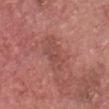Q: Was a biopsy performed?
A: no biopsy performed (imaged during a skin exam)
Q: Who is the patient?
A: male, in their 80s
Q: What is the imaging modality?
A: ~15 mm tile from a whole-body skin photo
Q: Lesion size?
A: ≈4.5 mm
Q: How was the tile lit?
A: white-light
Q: Lesion location?
A: the head or neck